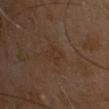notes: catalogued during a skin exam; not biopsied
lesion diameter: ~2.5 mm (longest diameter)
patient: male, aged around 60
anatomic site: the head or neck
image source: ~15 mm tile from a whole-body skin photo
automated metrics: a footprint of about 3.5 mm², an eccentricity of roughly 0.8, and a symmetry-axis asymmetry near 0.25